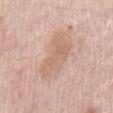lesion diameter: ~5.5 mm (longest diameter) | tile lighting: white-light | patient: male, in their 70s | image: ~15 mm crop, total-body skin-cancer survey | automated lesion analysis: a footprint of about 12 mm², an eccentricity of roughly 0.9, and a shape-asymmetry score of about 0.5 (0 = symmetric); a color-variation rating of about 2.5/10 and radial color variation of about 0.5; a nevus-likeness score of about 0/100 and a lesion-detection confidence of about 100/100 | location: the front of the torso.